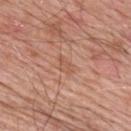Q: Was this lesion biopsied?
A: catalogued during a skin exam; not biopsied
Q: What are the patient's age and sex?
A: male, approximately 80 years of age
Q: What is the lesion's diameter?
A: about 2.5 mm
Q: Where on the body is the lesion?
A: the upper back
Q: How was this image acquired?
A: total-body-photography crop, ~15 mm field of view
Q: Automated lesion metrics?
A: a lesion area of about 3.5 mm², an outline eccentricity of about 0.8 (0 = round, 1 = elongated), and two-axis asymmetry of about 0.3; an average lesion color of about L≈56 a*≈23 b*≈31 (CIELAB) and a normalized border contrast of about 4.5; border irregularity of about 3 on a 0–10 scale, a color-variation rating of about 1/10, and radial color variation of about 0.5